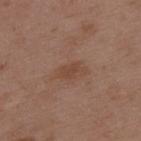follow-up = no biopsy performed (imaged during a skin exam) | tile lighting = white-light | acquisition = ~15 mm crop, total-body skin-cancer survey | location = the upper back | lesion size = ≈2.5 mm | patient = male, aged 48 to 52.